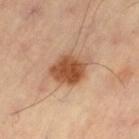automated lesion analysis = an eccentricity of roughly 0.65 and a symmetry-axis asymmetry near 0.15; a lesion color around L≈53 a*≈24 b*≈36 in CIELAB, about 15 CIELAB-L* units darker than the surrounding skin, and a normalized lesion–skin contrast near 10; a lesion-detection confidence of about 100/100 | tile lighting = cross-polarized illumination | location = the left thigh | imaging modality = ~15 mm crop, total-body skin-cancer survey | lesion diameter = ≈4.5 mm.